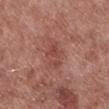Context: The tile uses white-light illumination. A male subject about 55 years old. The lesion-visualizer software estimated a lesion area of about 8.5 mm². And it measured a border-irregularity index near 2.5/10, a color-variation rating of about 3.5/10, and radial color variation of about 1. The analysis additionally found a classifier nevus-likeness of about 0/100 and a detector confidence of about 100 out of 100 that the crop contains a lesion. From the right lower leg. Cropped from a total-body skin-imaging series; the visible field is about 15 mm. The lesion's longest dimension is about 4 mm.